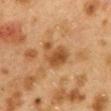| feature | finding |
|---|---|
| workup | total-body-photography surveillance lesion; no biopsy |
| anatomic site | the mid back |
| tile lighting | cross-polarized |
| diameter | ~4 mm (longest diameter) |
| image | total-body-photography crop, ~15 mm field of view |
| patient | female, aged 38–42 |